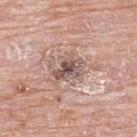Imaged during a routine full-body skin examination; the lesion was not biopsied and no histopathology is available.
Cropped from a whole-body photographic skin survey; the tile spans about 15 mm.
Located on the upper back.
Approximately 3.5 mm at its widest.
A female patient, aged approximately 65.
Automated tile analysis of the lesion measured a footprint of about 7.5 mm² and an outline eccentricity of about 0.7 (0 = round, 1 = elongated). The analysis additionally found roughly 12 lightness units darker than nearby skin and a lesion-to-skin contrast of about 8 (normalized; higher = more distinct). It also reported a within-lesion color-variation index near 6/10 and peripheral color asymmetry of about 1.5.
Imaged with white-light lighting.